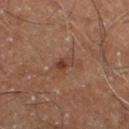The lesion was tiled from a total-body skin photograph and was not biopsied. A male patient aged 58 to 62. From the right thigh. Automated tile analysis of the lesion measured an area of roughly 3 mm² and two-axis asymmetry of about 0.35. The analysis additionally found an average lesion color of about L≈35 a*≈19 b*≈25 (CIELAB), a lesion–skin lightness drop of about 7, and a normalized border contrast of about 7. A roughly 15 mm field-of-view crop from a total-body skin photograph.